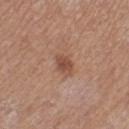anatomic site: the right lower leg; image source: 15 mm crop, total-body photography; patient: female, in their mid-40s; tile lighting: white-light.